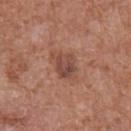Imaged during a routine full-body skin examination; the lesion was not biopsied and no histopathology is available. The patient is a male approximately 65 years of age. A roughly 15 mm field-of-view crop from a total-body skin photograph. The lesion is on the abdomen. Imaged with white-light lighting. Measured at roughly 3 mm in maximum diameter. An algorithmic analysis of the crop reported a footprint of about 6 mm², an outline eccentricity of about 0.55 (0 = round, 1 = elongated), and two-axis asymmetry of about 0.35.